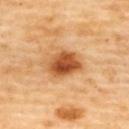biopsy status — imaged on a skin check; not biopsied
body site — the upper back
patient — female, about 60 years old
diameter — ~4 mm (longest diameter)
image source — 15 mm crop, total-body photography
illumination — cross-polarized illumination